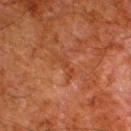biopsy status — imaged on a skin check; not biopsied | location — the left lower leg | imaging modality — ~15 mm tile from a whole-body skin photo | patient — male, aged 78–82 | diameter — ≈3.5 mm.